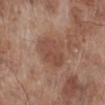biopsy status: catalogued during a skin exam; not biopsied | size: about 5.5 mm | image-analysis metrics: a footprint of about 12 mm², an eccentricity of roughly 0.8, and a shape-asymmetry score of about 0.25 (0 = symmetric); a classifier nevus-likeness of about 30/100 | illumination: white-light illumination | imaging modality: 15 mm crop, total-body photography | body site: the leg | subject: male, roughly 70 years of age.